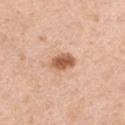Recorded during total-body skin imaging; not selected for excision or biopsy.
Automated image analysis of the tile measured a classifier nevus-likeness of about 95/100.
Approximately 3.5 mm at its widest.
Located on the arm.
A male patient, approximately 40 years of age.
Captured under white-light illumination.
A 15 mm close-up extracted from a 3D total-body photography capture.